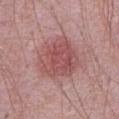Case summary:
- workup · no biopsy performed (imaged during a skin exam)
- diameter · ≈5.5 mm
- lighting · white-light illumination
- subject · male, about 70 years old
- image source · total-body-photography crop, ~15 mm field of view
- anatomic site · the front of the torso